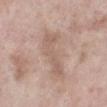This lesion was catalogued during total-body skin photography and was not selected for biopsy. The patient is a female aged 68 to 72. A lesion tile, about 15 mm wide, cut from a 3D total-body photograph. Automated image analysis of the tile measured an area of roughly 11 mm², an eccentricity of roughly 0.9, and a symmetry-axis asymmetry near 0.45. It also reported a mean CIELAB color near L≈59 a*≈17 b*≈25, roughly 7 lightness units darker than nearby skin, and a normalized border contrast of about 5. The lesion's longest dimension is about 5.5 mm. The lesion is located on the left lower leg.